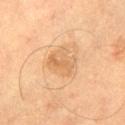follow-up: imaged on a skin check; not biopsied | lighting: cross-polarized illumination | lesion diameter: ~3.5 mm (longest diameter) | anatomic site: the right thigh | automated lesion analysis: a lesion area of about 8.5 mm² and a shape-asymmetry score of about 0.3 (0 = symmetric); a border-irregularity index near 3/10, internal color variation of about 4 on a 0–10 scale, and a peripheral color-asymmetry measure near 1.5; an automated nevus-likeness rating near 5 out of 100 and a detector confidence of about 100 out of 100 that the crop contains a lesion | patient: male, aged 58 to 62 | acquisition: ~15 mm tile from a whole-body skin photo.